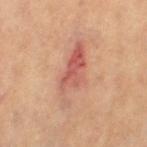  site: left thigh
  image:
    source: total-body photography crop
    field_of_view_mm: 15
  automated_metrics:
    area_mm2_approx: 13.0
    eccentricity: 0.95
    border_irregularity_0_10: 5.5
    color_variation_0_10: 6.0
    peripheral_color_asymmetry: 2.0
    nevus_likeness_0_100: 0
    lesion_detection_confidence_0_100: 100
  lighting: cross-polarized
  patient:
    sex: female
    age_approx: 65